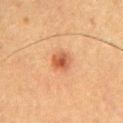{"biopsy_status": "not biopsied; imaged during a skin examination", "site": "chest", "lighting": "cross-polarized", "lesion_size": {"long_diameter_mm_approx": 3.0}, "image": {"source": "total-body photography crop", "field_of_view_mm": 15}, "patient": {"sex": "male", "age_approx": 50}}